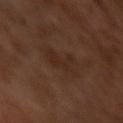follow-up: catalogued during a skin exam; not biopsied
imaging modality: total-body-photography crop, ~15 mm field of view
location: the arm
diameter: about 4 mm
subject: male, aged 28 to 32